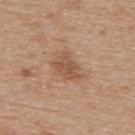Impression: Imaged during a routine full-body skin examination; the lesion was not biopsied and no histopathology is available. Background: The lesion is on the back. A female subject aged approximately 65. A region of skin cropped from a whole-body photographic capture, roughly 15 mm wide. Longest diameter approximately 4 mm. The total-body-photography lesion software estimated a footprint of about 8 mm², an eccentricity of roughly 0.75, and two-axis asymmetry of about 0.3. Imaged with white-light lighting.